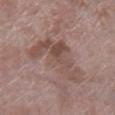notes: catalogued during a skin exam; not biopsied
acquisition: 15 mm crop, total-body photography
patient: female, aged approximately 70
anatomic site: the left lower leg